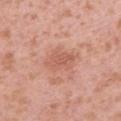<record>
  <biopsy_status>not biopsied; imaged during a skin examination</biopsy_status>
  <automated_metrics>
    <area_mm2_approx>6.0</area_mm2_approx>
    <shape_asymmetry>0.25</shape_asymmetry>
    <cielab_L>58</cielab_L>
    <cielab_a>26</cielab_a>
    <cielab_b>30</cielab_b>
    <vs_skin_darker_L>8.0</vs_skin_darker_L>
  </automated_metrics>
  <lesion_size>
    <long_diameter_mm_approx>3.5</long_diameter_mm_approx>
  </lesion_size>
  <patient>
    <sex>female</sex>
    <age_approx>40</age_approx>
  </patient>
  <lighting>white-light</lighting>
  <site>right upper arm</site>
  <image>
    <source>total-body photography crop</source>
    <field_of_view_mm>15</field_of_view_mm>
  </image>
</record>